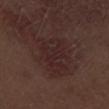Background:
Located on the left thigh. The patient is a male approximately 70 years of age. The tile uses white-light illumination. Longest diameter approximately 5 mm. The lesion-visualizer software estimated an area of roughly 14 mm², an eccentricity of roughly 0.8, and a symmetry-axis asymmetry near 0.35. The analysis additionally found a mean CIELAB color near L≈24 a*≈18 b*≈16, a lesion–skin lightness drop of about 5, and a normalized lesion–skin contrast near 5.5. A 15 mm crop from a total-body photograph taken for skin-cancer surveillance.